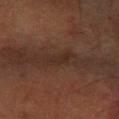  image:
    source: total-body photography crop
    field_of_view_mm: 15
  site: left forearm
  lighting: cross-polarized
  patient:
    sex: male
    age_approx: 65
  lesion_size:
    long_diameter_mm_approx: 3.0